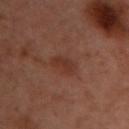No biopsy was performed on this lesion — it was imaged during a full skin examination and was not determined to be concerning. Automated image analysis of the tile measured a lesion–skin lightness drop of about 6. The analysis additionally found a border-irregularity rating of about 2.5/10, a within-lesion color-variation index near 1/10, and a peripheral color-asymmetry measure near 0.5. And it measured an automated nevus-likeness rating near 10 out of 100 and a lesion-detection confidence of about 100/100. This image is a 15 mm lesion crop taken from a total-body photograph. The patient is a female aged 53 to 57. The lesion is located on the chest. The recorded lesion diameter is about 3 mm.